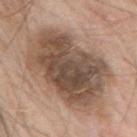Assessment:
Recorded during total-body skin imaging; not selected for excision or biopsy.
Image and clinical context:
This is a white-light tile. Automated image analysis of the tile measured an area of roughly 55 mm², an eccentricity of roughly 0.75, and a symmetry-axis asymmetry near 0.15. The analysis additionally found a border-irregularity rating of about 2.5/10, a within-lesion color-variation index near 7/10, and peripheral color asymmetry of about 2. From the right upper arm. Cropped from a total-body skin-imaging series; the visible field is about 15 mm. A male patient aged around 60. Approximately 10.5 mm at its widest.